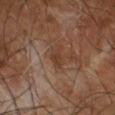biopsy_status: not biopsied; imaged during a skin examination
site: left forearm
image:
  source: total-body photography crop
  field_of_view_mm: 15
patient:
  sex: male
  age_approx: 55
lesion_size:
  long_diameter_mm_approx: 3.0